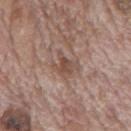biopsy_status: not biopsied; imaged during a skin examination
lighting: white-light
site: mid back
lesion_size:
  long_diameter_mm_approx: 3.5
patient:
  sex: male
  age_approx: 70
automated_metrics:
  area_mm2_approx: 6.0
  eccentricity: 0.45
  shape_asymmetry: 0.35
  vs_skin_darker_L: 9.0
  vs_skin_contrast_norm: 6.5
  color_variation_0_10: 3.5
  peripheral_color_asymmetry: 1.0
image:
  source: total-body photography crop
  field_of_view_mm: 15